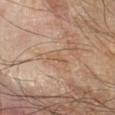No biopsy was performed on this lesion — it was imaged during a full skin examination and was not determined to be concerning.
An algorithmic analysis of the crop reported a footprint of about 3.5 mm², an eccentricity of roughly 0.85, and a symmetry-axis asymmetry near 0.5. The software also gave a border-irregularity index near 5/10 and internal color variation of about 1 on a 0–10 scale.
Cropped from a total-body skin-imaging series; the visible field is about 15 mm.
The lesion is on the right forearm.
Longest diameter approximately 3 mm.
This is a cross-polarized tile.